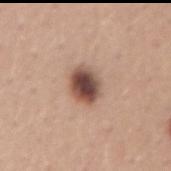biopsy status: no biopsy performed (imaged during a skin exam)
anatomic site: the lower back
acquisition: ~15 mm crop, total-body skin-cancer survey
automated metrics: internal color variation of about 7 on a 0–10 scale
illumination: white-light illumination
lesion diameter: about 3.5 mm
subject: female, aged around 50